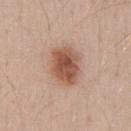Assessment:
No biopsy was performed on this lesion — it was imaged during a full skin examination and was not determined to be concerning.
Background:
Automated image analysis of the tile measured an area of roughly 14 mm² and a symmetry-axis asymmetry near 0.2. The analysis additionally found a classifier nevus-likeness of about 100/100 and lesion-presence confidence of about 100/100. A male patient, approximately 30 years of age. The lesion's longest dimension is about 4.5 mm. The lesion is located on the front of the torso. A close-up tile cropped from a whole-body skin photograph, about 15 mm across.